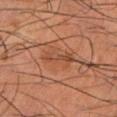Part of a total-body skin-imaging series; this lesion was reviewed on a skin check and was not flagged for biopsy. Measured at roughly 5 mm in maximum diameter. From the left lower leg. A male patient, aged around 55. Captured under cross-polarized illumination. A region of skin cropped from a whole-body photographic capture, roughly 15 mm wide. An algorithmic analysis of the crop reported a border-irregularity rating of about 4.5/10 and radial color variation of about 1.5. And it measured lesion-presence confidence of about 90/100.